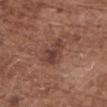Clinical impression: This lesion was catalogued during total-body skin photography and was not selected for biopsy. Image and clinical context: The lesion-visualizer software estimated about 8 CIELAB-L* units darker than the surrounding skin and a lesion-to-skin contrast of about 7 (normalized; higher = more distinct). A region of skin cropped from a whole-body photographic capture, roughly 15 mm wide. About 3.5 mm across. The tile uses white-light illumination. Located on the upper back. A female patient aged 73–77.